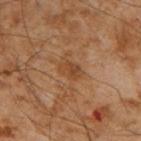No biopsy was performed on this lesion — it was imaged during a full skin examination and was not determined to be concerning. The subject is a male in their mid- to late 50s. A roughly 15 mm field-of-view crop from a total-body skin photograph. The lesion is on the right upper arm.